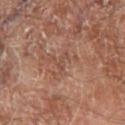Assessment:
Captured during whole-body skin photography for melanoma surveillance; the lesion was not biopsied.
Acquisition and patient details:
A 15 mm close-up extracted from a 3D total-body photography capture. The patient is a male aged 68–72. The lesion is located on the right thigh. The lesion-visualizer software estimated a symmetry-axis asymmetry near 0.45. And it measured border irregularity of about 5 on a 0–10 scale, a color-variation rating of about 0/10, and radial color variation of about 0.